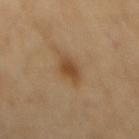The lesion was photographed on a routine skin check and not biopsied; there is no pathology result. A male subject, roughly 70 years of age. A 15 mm close-up extracted from a 3D total-body photography capture. The lesion is on the back. Automated tile analysis of the lesion measured a footprint of about 7 mm², an outline eccentricity of about 0.85 (0 = round, 1 = elongated), and two-axis asymmetry of about 0.15. The software also gave a mean CIELAB color near L≈44 a*≈16 b*≈33. It also reported a nevus-likeness score of about 75/100 and a lesion-detection confidence of about 100/100.